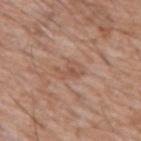  biopsy_status: not biopsied; imaged during a skin examination
  lesion_size:
    long_diameter_mm_approx: 3.5
  site: chest
  image:
    source: total-body photography crop
    field_of_view_mm: 15
  patient:
    sex: male
    age_approx: 60
  lighting: white-light